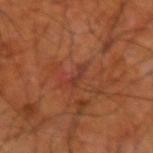| field | value |
|---|---|
| follow-up | catalogued during a skin exam; not biopsied |
| patient | male, approximately 65 years of age |
| lighting | cross-polarized |
| site | the right upper arm |
| imaging modality | ~15 mm tile from a whole-body skin photo |
| lesion size | ~3.5 mm (longest diameter) |